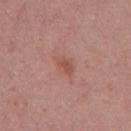Clinical impression:
Part of a total-body skin-imaging series; this lesion was reviewed on a skin check and was not flagged for biopsy.
Context:
This is a white-light tile. Measured at roughly 2.5 mm in maximum diameter. The lesion is located on the right upper arm. A 15 mm crop from a total-body photograph taken for skin-cancer surveillance. A female patient, aged 48 to 52. The lesion-visualizer software estimated a symmetry-axis asymmetry near 0.2. It also reported an automated nevus-likeness rating near 5 out of 100 and a detector confidence of about 100 out of 100 that the crop contains a lesion.